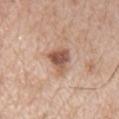Impression: Recorded during total-body skin imaging; not selected for excision or biopsy. Clinical summary: A male subject aged 63 to 67. Automated tile analysis of the lesion measured a lesion area of about 7.5 mm². The analysis additionally found a border-irregularity index near 4/10 and a peripheral color-asymmetry measure near 1.5. A 15 mm close-up extracted from a 3D total-body photography capture. Located on the chest. About 3.5 mm across. The tile uses white-light illumination.